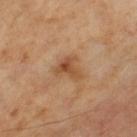workup: no biopsy performed (imaged during a skin exam) | image source: 15 mm crop, total-body photography | patient: male, aged approximately 65.